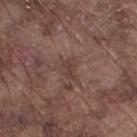  biopsy_status: not biopsied; imaged during a skin examination
  image:
    source: total-body photography crop
    field_of_view_mm: 15
  site: right thigh
  lesion_size:
    long_diameter_mm_approx: 2.5
  lighting: white-light
  patient:
    sex: male
    age_approx: 75